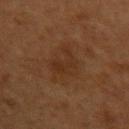* notes — total-body-photography surveillance lesion; no biopsy
* body site — the upper back
* diameter — about 4 mm
* patient — female, about 40 years old
* image source — ~15 mm crop, total-body skin-cancer survey
* automated lesion analysis — an area of roughly 6 mm², an eccentricity of roughly 0.8, and a shape-asymmetry score of about 0.6 (0 = symmetric); a border-irregularity rating of about 7/10, a within-lesion color-variation index near 2.5/10, and a peripheral color-asymmetry measure near 1